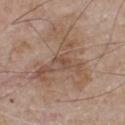Q: Was a biopsy performed?
A: total-body-photography surveillance lesion; no biopsy